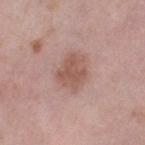The lesion was photographed on a routine skin check and not biopsied; there is no pathology result. A lesion tile, about 15 mm wide, cut from a 3D total-body photograph. On the leg. A female patient, aged 38 to 42.